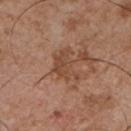Part of a total-body skin-imaging series; this lesion was reviewed on a skin check and was not flagged for biopsy. The lesion's longest dimension is about 4.5 mm. From the chest. The subject is a male aged around 55. A 15 mm close-up extracted from a 3D total-body photography capture. This is a white-light tile.